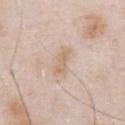workup: total-body-photography surveillance lesion; no biopsy | acquisition: ~15 mm tile from a whole-body skin photo | subject: male, aged 53 to 57 | lighting: white-light | body site: the chest | lesion size: ~4 mm (longest diameter).